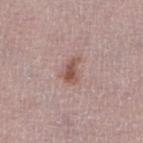The lesion was tiled from a total-body skin photograph and was not biopsied.
The patient is a female about 50 years old.
The lesion is located on the left lower leg.
Automated tile analysis of the lesion measured a shape eccentricity near 0.8 and a shape-asymmetry score of about 0.35 (0 = symmetric). And it measured internal color variation of about 2.5 on a 0–10 scale. It also reported a nevus-likeness score of about 75/100 and a lesion-detection confidence of about 100/100.
The recorded lesion diameter is about 3 mm.
Cropped from a total-body skin-imaging series; the visible field is about 15 mm.